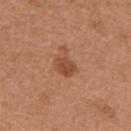Notes:
– follow-up — no biopsy performed (imaged during a skin exam)
– diameter — ~3 mm (longest diameter)
– location — the back
– imaging modality — total-body-photography crop, ~15 mm field of view
– illumination — white-light
– subject — female, approximately 40 years of age